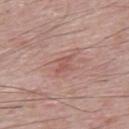Imaged during a routine full-body skin examination; the lesion was not biopsied and no histopathology is available. A male patient aged around 65. The lesion is located on the back. The lesion's longest dimension is about 2.5 mm. Imaged with white-light lighting. A lesion tile, about 15 mm wide, cut from a 3D total-body photograph. An algorithmic analysis of the crop reported an area of roughly 2.5 mm² and an outline eccentricity of about 0.9 (0 = round, 1 = elongated). And it measured a lesion color around L≈54 a*≈24 b*≈25 in CIELAB, a lesion–skin lightness drop of about 7, and a lesion-to-skin contrast of about 5.5 (normalized; higher = more distinct). And it measured border irregularity of about 5.5 on a 0–10 scale and a color-variation rating of about 0/10.